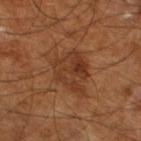Captured during whole-body skin photography for melanoma surveillance; the lesion was not biopsied. The lesion is located on the left lower leg. A male subject aged 63 to 67. An algorithmic analysis of the crop reported a footprint of about 18 mm², a shape eccentricity near 0.65, and a symmetry-axis asymmetry near 0.35. The analysis additionally found a lesion color around L≈36 a*≈22 b*≈31 in CIELAB, a lesion–skin lightness drop of about 7, and a normalized border contrast of about 6.5. It also reported a nevus-likeness score of about 5/100 and a lesion-detection confidence of about 100/100. This is a cross-polarized tile. This image is a 15 mm lesion crop taken from a total-body photograph. Approximately 6 mm at its widest.